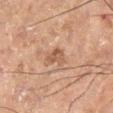Q: Was this lesion biopsied?
A: catalogued during a skin exam; not biopsied
Q: Illumination type?
A: cross-polarized
Q: How was this image acquired?
A: ~15 mm tile from a whole-body skin photo
Q: How large is the lesion?
A: ≈3 mm
Q: Patient demographics?
A: male, approximately 60 years of age
Q: Where on the body is the lesion?
A: the left lower leg